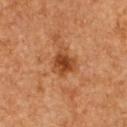No biopsy was performed on this lesion — it was imaged during a full skin examination and was not determined to be concerning. From the upper back. The subject is a female approximately 60 years of age. Captured under cross-polarized illumination. An algorithmic analysis of the crop reported an area of roughly 7 mm², a shape eccentricity near 0.4, and two-axis asymmetry of about 0.35. The analysis additionally found a nevus-likeness score of about 75/100 and lesion-presence confidence of about 100/100. A roughly 15 mm field-of-view crop from a total-body skin photograph. The lesion's longest dimension is about 3.5 mm.